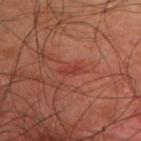Assessment:
Captured during whole-body skin photography for melanoma surveillance; the lesion was not biopsied.
Image and clinical context:
Cropped from a total-body skin-imaging series; the visible field is about 15 mm. A male patient roughly 45 years of age. This is a cross-polarized tile. Measured at roughly 2 mm in maximum diameter. The lesion is located on the upper back.